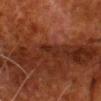No biopsy was performed on this lesion — it was imaged during a full skin examination and was not determined to be concerning. The patient is a male aged 78–82. Measured at roughly 2.5 mm in maximum diameter. Captured under cross-polarized illumination. Cropped from a total-body skin-imaging series; the visible field is about 15 mm. The lesion is on the head or neck. Automated image analysis of the tile measured about 5 CIELAB-L* units darker than the surrounding skin.